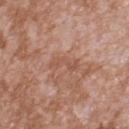Imaged during a routine full-body skin examination; the lesion was not biopsied and no histopathology is available.
A region of skin cropped from a whole-body photographic capture, roughly 15 mm wide.
The lesion's longest dimension is about 3.5 mm.
Imaged with white-light lighting.
Located on the back.
The subject is a male roughly 45 years of age.